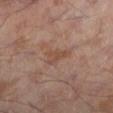<lesion>
<biopsy_status>not biopsied; imaged during a skin examination</biopsy_status>
<image>
  <source>total-body photography crop</source>
  <field_of_view_mm>15</field_of_view_mm>
</image>
<lighting>cross-polarized</lighting>
<lesion_size>
  <long_diameter_mm_approx>3.5</long_diameter_mm_approx>
</lesion_size>
<patient>
  <sex>male</sex>
  <age_approx>55</age_approx>
</patient>
<automated_metrics>
  <eccentricity>0.8</eccentricity>
  <shape_asymmetry>0.4</shape_asymmetry>
  <cielab_L>44</cielab_L>
  <cielab_a>18</cielab_a>
  <cielab_b>25</cielab_b>
  <vs_skin_darker_L>6.0</vs_skin_darker_L>
  <vs_skin_contrast_norm>5.5</vs_skin_contrast_norm>
  <nevus_likeness_0_100>0</nevus_likeness_0_100>
  <lesion_detection_confidence_0_100>100</lesion_detection_confidence_0_100>
</automated_metrics>
<site>left lower leg</site>
</lesion>